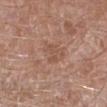The lesion was photographed on a routine skin check and not biopsied; there is no pathology result. Measured at roughly 3.5 mm in maximum diameter. The lesion is on the left lower leg. A roughly 15 mm field-of-view crop from a total-body skin photograph. The subject is a male aged approximately 60.